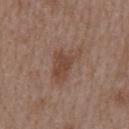Imaged during a routine full-body skin examination; the lesion was not biopsied and no histopathology is available. A female subject approximately 45 years of age. A 15 mm close-up extracted from a 3D total-body photography capture. From the upper back.